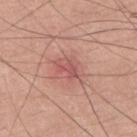Q: Was a biopsy performed?
A: no biopsy performed (imaged during a skin exam)
Q: What lighting was used for the tile?
A: white-light illumination
Q: What is the lesion's diameter?
A: ≈3 mm
Q: What did automated image analysis measure?
A: a footprint of about 4 mm², a shape eccentricity near 0.8, and two-axis asymmetry of about 0.35
Q: What are the patient's age and sex?
A: male, aged 23–27
Q: What is the imaging modality?
A: ~15 mm tile from a whole-body skin photo
Q: What is the anatomic site?
A: the back